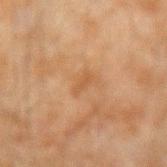Part of a total-body skin-imaging series; this lesion was reviewed on a skin check and was not flagged for biopsy. A 15 mm crop from a total-body photograph taken for skin-cancer surveillance. The lesion is on the arm. The subject is a male aged approximately 45.